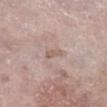Clinical impression: Part of a total-body skin-imaging series; this lesion was reviewed on a skin check and was not flagged for biopsy. Context: The tile uses white-light illumination. Approximately 2.5 mm at its widest. Located on the right lower leg. A 15 mm close-up tile from a total-body photography series done for melanoma screening. A female patient, approximately 70 years of age. Automated tile analysis of the lesion measured internal color variation of about 0 on a 0–10 scale and radial color variation of about 0. It also reported a classifier nevus-likeness of about 0/100.